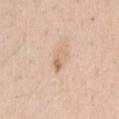Recorded during total-body skin imaging; not selected for excision or biopsy.
Imaged with white-light lighting.
A region of skin cropped from a whole-body photographic capture, roughly 15 mm wide.
Approximately 2.5 mm at its widest.
The lesion is located on the right upper arm.
A female subject aged 38–42.